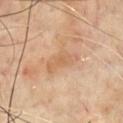biopsy status — imaged on a skin check; not biopsied | diameter — ~4 mm (longest diameter) | automated metrics — a lesion area of about 6 mm² and two-axis asymmetry of about 0.4; an average lesion color of about L≈59 a*≈19 b*≈34 (CIELAB), a lesion–skin lightness drop of about 7, and a normalized border contrast of about 5.5; border irregularity of about 4.5 on a 0–10 scale and a within-lesion color-variation index near 2/10; a nevus-likeness score of about 0/100 and a detector confidence of about 100 out of 100 that the crop contains a lesion | body site — the chest | patient — male, aged 68–72 | image source — ~15 mm crop, total-body skin-cancer survey | tile lighting — cross-polarized.